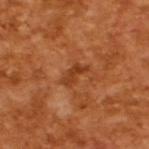Recorded during total-body skin imaging; not selected for excision or biopsy.
A male subject, in their mid-60s.
A lesion tile, about 15 mm wide, cut from a 3D total-body photograph.